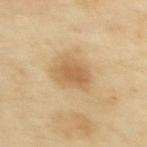Clinical impression:
Imaged during a routine full-body skin examination; the lesion was not biopsied and no histopathology is available.
Acquisition and patient details:
A roughly 15 mm field-of-view crop from a total-body skin photograph. The lesion's longest dimension is about 3.5 mm. The subject is a female aged 43–47. The lesion-visualizer software estimated lesion-presence confidence of about 100/100. Located on the upper back. The tile uses cross-polarized illumination.